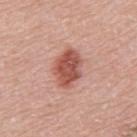| feature | finding |
|---|---|
| follow-up | total-body-photography surveillance lesion; no biopsy |
| diameter | about 4.5 mm |
| acquisition | total-body-photography crop, ~15 mm field of view |
| body site | the back |
| automated lesion analysis | an area of roughly 11 mm², an outline eccentricity of about 0.75 (0 = round, 1 = elongated), and a symmetry-axis asymmetry near 0.15; a normalized lesion–skin contrast near 9.5; a border-irregularity index near 1.5/10, a color-variation rating of about 4/10, and a peripheral color-asymmetry measure near 1.5; a classifier nevus-likeness of about 95/100 and a detector confidence of about 100 out of 100 that the crop contains a lesion |
| subject | male, aged approximately 70 |